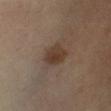Notes:
* image source · ~15 mm crop, total-body skin-cancer survey
* patient · male, approximately 65 years of age
* site · the left lower leg
* lesion size · ~3.5 mm (longest diameter)